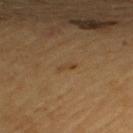Clinical impression:
Captured during whole-body skin photography for melanoma surveillance; the lesion was not biopsied.
Clinical summary:
A close-up tile cropped from a whole-body skin photograph, about 15 mm across. Captured under cross-polarized illumination. The subject is a male aged 83 or older. Located on the back.